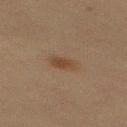Clinical impression: This lesion was catalogued during total-body skin photography and was not selected for biopsy. Clinical summary: The lesion is on the mid back. A male patient aged approximately 60. Approximately 3 mm at its widest. A roughly 15 mm field-of-view crop from a total-body skin photograph.